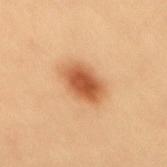biopsy status: no biopsy performed (imaged during a skin exam)
diameter: ~4.5 mm (longest diameter)
location: the back
tile lighting: cross-polarized illumination
automated metrics: an eccentricity of roughly 0.75 and a shape-asymmetry score of about 0.15 (0 = symmetric); roughly 15 lightness units darker than nearby skin and a lesion-to-skin contrast of about 9.5 (normalized; higher = more distinct); a lesion-detection confidence of about 100/100
imaging modality: 15 mm crop, total-body photography
patient: female, about 25 years old